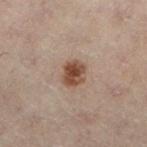Captured during whole-body skin photography for melanoma surveillance; the lesion was not biopsied.
A lesion tile, about 15 mm wide, cut from a 3D total-body photograph.
The patient is a female aged 53 to 57.
From the left lower leg.
Automated image analysis of the tile measured a footprint of about 6 mm², an eccentricity of roughly 0.55, and two-axis asymmetry of about 0.15. The software also gave a nevus-likeness score of about 95/100 and lesion-presence confidence of about 100/100.
Approximately 3 mm at its widest.